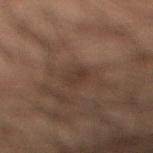Impression:
Captured during whole-body skin photography for melanoma surveillance; the lesion was not biopsied.
Acquisition and patient details:
The lesion is on the right lower leg. The lesion-visualizer software estimated a normalized lesion–skin contrast near 6. The analysis additionally found peripheral color asymmetry of about 0.5. A male patient, aged 48–52. Cropped from a total-body skin-imaging series; the visible field is about 15 mm.